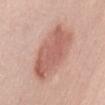{"biopsy_status": "not biopsied; imaged during a skin examination", "lesion_size": {"long_diameter_mm_approx": 8.0}, "patient": {"sex": "female", "age_approx": 50}, "site": "abdomen", "image": {"source": "total-body photography crop", "field_of_view_mm": 15}}